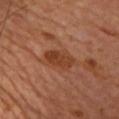<lesion>
<site>chest</site>
<patient>
  <sex>female</sex>
  <age_approx>60</age_approx>
</patient>
<image>
  <source>total-body photography crop</source>
  <field_of_view_mm>15</field_of_view_mm>
</image>
<lesion_size>
  <long_diameter_mm_approx>4.0</long_diameter_mm_approx>
</lesion_size>
<automated_metrics>
  <vs_skin_contrast_norm>8.5</vs_skin_contrast_norm>
  <border_irregularity_0_10>2.5</border_irregularity_0_10>
  <peripheral_color_asymmetry>1.5</peripheral_color_asymmetry>
</automated_metrics>
</lesion>